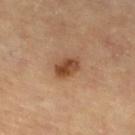This lesion was catalogued during total-body skin photography and was not selected for biopsy. A female patient roughly 70 years of age. Located on the leg. Approximately 3.5 mm at its widest. Captured under cross-polarized illumination. Cropped from a whole-body photographic skin survey; the tile spans about 15 mm.